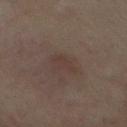biopsy_status: not biopsied; imaged during a skin examination
image:
  source: total-body photography crop
  field_of_view_mm: 15
lighting: cross-polarized
patient:
  sex: male
  age_approx: 35
site: back